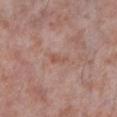| field | value |
|---|---|
| biopsy status | no biopsy performed (imaged during a skin exam) |
| automated metrics | a lesion area of about 2 mm², a shape eccentricity near 0.9, and a shape-asymmetry score of about 0.5 (0 = symmetric); a lesion color around L≈53 a*≈22 b*≈27 in CIELAB, a lesion–skin lightness drop of about 7, and a normalized border contrast of about 5.5; an automated nevus-likeness rating near 0 out of 100 and a lesion-detection confidence of about 100/100 |
| lesion diameter | ~2.5 mm (longest diameter) |
| anatomic site | the left lower leg |
| imaging modality | 15 mm crop, total-body photography |
| patient | male, aged 53 to 57 |
| illumination | white-light illumination |